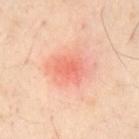{
  "biopsy_status": "not biopsied; imaged during a skin examination",
  "site": "chest",
  "automated_metrics": {
    "eccentricity": 0.6,
    "shape_asymmetry": 0.25,
    "border_irregularity_0_10": 2.0,
    "peripheral_color_asymmetry": 1.0,
    "nevus_likeness_0_100": 0,
    "lesion_detection_confidence_0_100": 100
  },
  "lesion_size": {
    "long_diameter_mm_approx": 3.5
  },
  "image": {
    "source": "total-body photography crop",
    "field_of_view_mm": 15
  },
  "patient": {
    "sex": "male",
    "age_approx": 55
  }
}